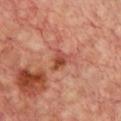Captured during whole-body skin photography for melanoma surveillance; the lesion was not biopsied.
Cropped from a whole-body photographic skin survey; the tile spans about 15 mm.
From the chest.
The total-body-photography lesion software estimated a footprint of about 4 mm², an outline eccentricity of about 0.65 (0 = round, 1 = elongated), and two-axis asymmetry of about 0.45. The analysis additionally found a lesion color around L≈47 a*≈28 b*≈31 in CIELAB and roughly 10 lightness units darker than nearby skin.
Longest diameter approximately 2.5 mm.
This is a cross-polarized tile.
The patient is a female aged around 45.